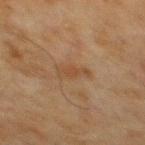Clinical impression: Part of a total-body skin-imaging series; this lesion was reviewed on a skin check and was not flagged for biopsy. Image and clinical context: A 15 mm close-up extracted from a 3D total-body photography capture. The lesion is located on the mid back. This is a cross-polarized tile. Automated image analysis of the tile measured a lesion–skin lightness drop of about 5 and a lesion-to-skin contrast of about 5.5 (normalized; higher = more distinct). It also reported border irregularity of about 4 on a 0–10 scale, internal color variation of about 1.5 on a 0–10 scale, and radial color variation of about 0.5. The analysis additionally found a nevus-likeness score of about 0/100 and a detector confidence of about 100 out of 100 that the crop contains a lesion. A male subject aged approximately 70.